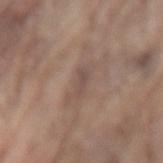Q: What did automated image analysis measure?
A: a nevus-likeness score of about 0/100
Q: What are the patient's age and sex?
A: male, aged 78–82
Q: Where on the body is the lesion?
A: the lower back
Q: How large is the lesion?
A: ≈2.5 mm
Q: What lighting was used for the tile?
A: white-light illumination
Q: How was this image acquired?
A: 15 mm crop, total-body photography